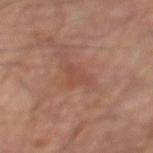This lesion was catalogued during total-body skin photography and was not selected for biopsy.
The lesion is located on the left lower leg.
The patient is a male aged around 70.
Cropped from a whole-body photographic skin survey; the tile spans about 15 mm.